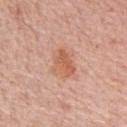Case summary:
* follow-up — catalogued during a skin exam; not biopsied
* size — about 3.5 mm
* anatomic site — the left upper arm
* patient — female, in their mid- to late 60s
* imaging modality — ~15 mm tile from a whole-body skin photo